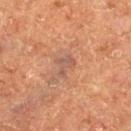Recorded during total-body skin imaging; not selected for excision or biopsy. The lesion is located on the right thigh. A male patient aged approximately 75. Cropped from a total-body skin-imaging series; the visible field is about 15 mm. This is a cross-polarized tile. The lesion-visualizer software estimated an average lesion color of about L≈43 a*≈18 b*≈24 (CIELAB), a lesion–skin lightness drop of about 6, and a normalized lesion–skin contrast near 6. It also reported a border-irregularity rating of about 5.5/10 and a color-variation rating of about 1.5/10.